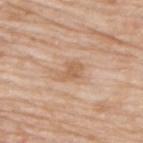Q: Is there a histopathology result?
A: no biopsy performed (imaged during a skin exam)
Q: Automated lesion metrics?
A: a lesion area of about 4 mm², an outline eccentricity of about 0.7 (0 = round, 1 = elongated), and a symmetry-axis asymmetry near 0.25; a border-irregularity index near 3/10 and radial color variation of about 0.5
Q: Who is the patient?
A: male, aged 63–67
Q: How was the tile lit?
A: white-light illumination
Q: What is the imaging modality?
A: ~15 mm crop, total-body skin-cancer survey
Q: Lesion location?
A: the upper back
Q: Lesion size?
A: ≈2.5 mm